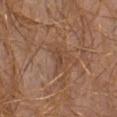* follow-up · total-body-photography surveillance lesion; no biopsy
* diameter · ≈3 mm
* subject · male, roughly 40 years of age
* image-analysis metrics · a shape eccentricity near 0.9 and a symmetry-axis asymmetry near 0.25; a color-variation rating of about 1/10 and a peripheral color-asymmetry measure near 0.5; an automated nevus-likeness rating near 0 out of 100 and lesion-presence confidence of about 80/100
* anatomic site · the abdomen
* imaging modality · 15 mm crop, total-body photography